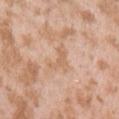Recorded during total-body skin imaging; not selected for excision or biopsy. A lesion tile, about 15 mm wide, cut from a 3D total-body photograph. The subject is a female aged around 25. The lesion is on the arm. This is a white-light tile. Automated tile analysis of the lesion measured an area of roughly 3.5 mm² and a shape-asymmetry score of about 0.6 (0 = symmetric). And it measured a lesion color around L≈63 a*≈20 b*≈33 in CIELAB, roughly 7 lightness units darker than nearby skin, and a lesion-to-skin contrast of about 5 (normalized; higher = more distinct). It also reported border irregularity of about 8 on a 0–10 scale, a within-lesion color-variation index near 0/10, and a peripheral color-asymmetry measure near 0. The analysis additionally found lesion-presence confidence of about 100/100. The recorded lesion diameter is about 3.5 mm.